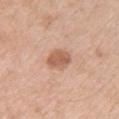{"biopsy_status": "not biopsied; imaged during a skin examination", "site": "front of the torso", "lighting": "white-light", "lesion_size": {"long_diameter_mm_approx": 3.0}, "image": {"source": "total-body photography crop", "field_of_view_mm": 15}, "patient": {"sex": "male", "age_approx": 65}, "automated_metrics": {"cielab_L": 60, "cielab_a": 23, "cielab_b": 32, "vs_skin_darker_L": 11.0, "color_variation_0_10": 2.5, "peripheral_color_asymmetry": 1.0}}